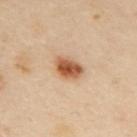Q: Was this lesion biopsied?
A: catalogued during a skin exam; not biopsied
Q: What kind of image is this?
A: 15 mm crop, total-body photography
Q: Lesion location?
A: the upper back
Q: How large is the lesion?
A: ≈3.5 mm
Q: Who is the patient?
A: female, in their 40s
Q: What lighting was used for the tile?
A: cross-polarized